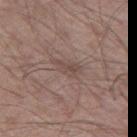Clinical impression: Recorded during total-body skin imaging; not selected for excision or biopsy. Background: Automated image analysis of the tile measured a border-irregularity rating of about 4/10 and peripheral color asymmetry of about 1. It also reported a nevus-likeness score of about 0/100 and lesion-presence confidence of about 95/100. A lesion tile, about 15 mm wide, cut from a 3D total-body photograph. A male subject aged around 55. The lesion is on the left thigh.